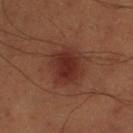Q: Was this lesion biopsied?
A: total-body-photography surveillance lesion; no biopsy
Q: What are the patient's age and sex?
A: male, approximately 50 years of age
Q: Lesion location?
A: the left lower leg
Q: What kind of image is this?
A: total-body-photography crop, ~15 mm field of view
Q: What is the lesion's diameter?
A: ≈4 mm
Q: What did automated image analysis measure?
A: a shape-asymmetry score of about 0.15 (0 = symmetric); a border-irregularity rating of about 1.5/10, internal color variation of about 3 on a 0–10 scale, and a peripheral color-asymmetry measure near 1
Q: Illumination type?
A: cross-polarized illumination